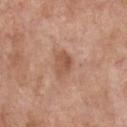Assessment: Part of a total-body skin-imaging series; this lesion was reviewed on a skin check and was not flagged for biopsy. Context: Captured under white-light illumination. Located on the front of the torso. The patient is a female aged 58–62. Approximately 3 mm at its widest. The total-body-photography lesion software estimated an outline eccentricity of about 0.65 (0 = round, 1 = elongated) and a shape-asymmetry score of about 0.35 (0 = symmetric). The analysis additionally found a border-irregularity index near 3/10, internal color variation of about 3 on a 0–10 scale, and radial color variation of about 1. It also reported a classifier nevus-likeness of about 5/100 and a detector confidence of about 100 out of 100 that the crop contains a lesion. This image is a 15 mm lesion crop taken from a total-body photograph.